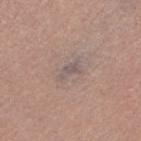Q: Was this lesion biopsied?
A: no biopsy performed (imaged during a skin exam)
Q: What is the imaging modality?
A: ~15 mm tile from a whole-body skin photo
Q: How was the tile lit?
A: white-light illumination
Q: How large is the lesion?
A: about 3 mm
Q: Who is the patient?
A: female, aged 58 to 62
Q: What did automated image analysis measure?
A: a mean CIELAB color near L≈54 a*≈13 b*≈17, roughly 7 lightness units darker than nearby skin, and a normalized lesion–skin contrast near 6.5; a nevus-likeness score of about 0/100 and lesion-presence confidence of about 70/100
Q: Where on the body is the lesion?
A: the right thigh